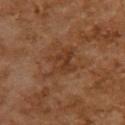Captured during whole-body skin photography for melanoma surveillance; the lesion was not biopsied.
The lesion-visualizer software estimated a lesion color around L≈31 a*≈19 b*≈28 in CIELAB and a lesion–skin lightness drop of about 6. And it measured a lesion-detection confidence of about 100/100.
The subject is a female approximately 60 years of age.
On the upper back.
Captured under cross-polarized illumination.
About 4.5 mm across.
This image is a 15 mm lesion crop taken from a total-body photograph.